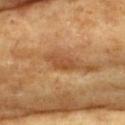• lesion size — ≈3.5 mm
• lighting — cross-polarized illumination
• location — the front of the torso
• image source — ~15 mm crop, total-body skin-cancer survey
• subject — female, in their mid- to late 70s
• automated lesion analysis — a lesion area of about 4.5 mm², an eccentricity of roughly 0.9, and two-axis asymmetry of about 0.3; a classifier nevus-likeness of about 0/100 and lesion-presence confidence of about 90/100